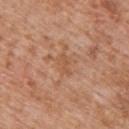Captured during whole-body skin photography for melanoma surveillance; the lesion was not biopsied. This is a white-light tile. About 2.5 mm across. The subject is a male aged 58–62. The lesion is located on the back. Cropped from a total-body skin-imaging series; the visible field is about 15 mm. An algorithmic analysis of the crop reported a border-irregularity index near 3/10, a within-lesion color-variation index near 0/10, and peripheral color asymmetry of about 0.